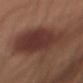Findings:
* notes — total-body-photography surveillance lesion; no biopsy
* tile lighting — white-light
* anatomic site — the left upper arm
* acquisition — 15 mm crop, total-body photography
* lesion size — ~11.5 mm (longest diameter)
* automated lesion analysis — a symmetry-axis asymmetry near 0.25
* subject — male, aged 53–57